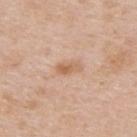workup = total-body-photography surveillance lesion; no biopsy
subject = male, in their 50s
image-analysis metrics = a lesion area of about 4 mm² and a shape eccentricity near 0.9; border irregularity of about 3 on a 0–10 scale, a within-lesion color-variation index near 3/10, and radial color variation of about 1
illumination = white-light illumination
site = the upper back
imaging modality = ~15 mm crop, total-body skin-cancer survey